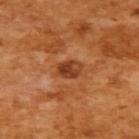<record>
<biopsy_status>not biopsied; imaged during a skin examination</biopsy_status>
<image>
  <source>total-body photography crop</source>
  <field_of_view_mm>15</field_of_view_mm>
</image>
<lighting>cross-polarized</lighting>
<patient>
  <sex>female</sex>
  <age_approx>55</age_approx>
</patient>
<lesion_size>
  <long_diameter_mm_approx>3.0</long_diameter_mm_approx>
</lesion_size>
<site>upper back</site>
<automated_metrics>
  <eccentricity>0.7</eccentricity>
  <shape_asymmetry>0.2</shape_asymmetry>
  <border_irregularity_0_10>2.0</border_irregularity_0_10>
  <color_variation_0_10>5.5</color_variation_0_10>
  <peripheral_color_asymmetry>2.0</peripheral_color_asymmetry>
</automated_metrics>
</record>